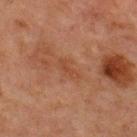workup=imaged on a skin check; not biopsied
patient=male, roughly 65 years of age
body site=the front of the torso
automated metrics=a lesion area of about 3 mm², a shape eccentricity near 0.95, and a symmetry-axis asymmetry near 0.45; border irregularity of about 5 on a 0–10 scale and peripheral color asymmetry of about 0; a nevus-likeness score of about 0/100
imaging modality=total-body-photography crop, ~15 mm field of view
tile lighting=cross-polarized
size=about 3 mm